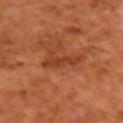notes: no biopsy performed (imaged during a skin exam); anatomic site: the left upper arm; diameter: ≈4 mm; patient: male, aged 58–62; illumination: cross-polarized; imaging modality: ~15 mm tile from a whole-body skin photo.